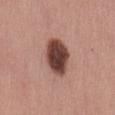biopsy_status: not biopsied; imaged during a skin examination
image:
  source: total-body photography crop
  field_of_view_mm: 15
site: back
patient:
  sex: female
  age_approx: 55
automated_metrics:
  nevus_likeness_0_100: 75
  lesion_detection_confidence_0_100: 100